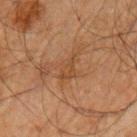Part of a total-body skin-imaging series; this lesion was reviewed on a skin check and was not flagged for biopsy. A male patient aged 63–67. The lesion is on the left upper arm. Cropped from a whole-body photographic skin survey; the tile spans about 15 mm.